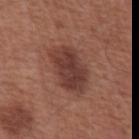Notes:
- biopsy status: imaged on a skin check; not biopsied
- lesion diameter: ≈6 mm
- illumination: white-light illumination
- body site: the abdomen
- subject: male, approximately 65 years of age
- image: total-body-photography crop, ~15 mm field of view